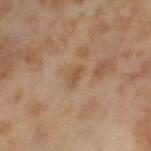Part of a total-body skin-imaging series; this lesion was reviewed on a skin check and was not flagged for biopsy.
The lesion-visualizer software estimated a lesion area of about 2.5 mm², an outline eccentricity of about 0.85 (0 = round, 1 = elongated), and a symmetry-axis asymmetry near 0.45. It also reported a border-irregularity index near 4.5/10 and peripheral color asymmetry of about 0. The analysis additionally found a classifier nevus-likeness of about 0/100 and a lesion-detection confidence of about 100/100.
Approximately 2.5 mm at its widest.
This image is a 15 mm lesion crop taken from a total-body photograph.
The lesion is on the left thigh.
A female patient, approximately 55 years of age.
The tile uses cross-polarized illumination.